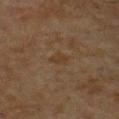{
  "biopsy_status": "not biopsied; imaged during a skin examination",
  "site": "chest",
  "patient": {
    "sex": "male",
    "age_approx": 60
  },
  "lesion_size": {
    "long_diameter_mm_approx": 2.5
  },
  "image": {
    "source": "total-body photography crop",
    "field_of_view_mm": 15
  }
}